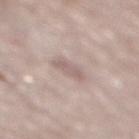patient: male, in their 70s
lesion size: ≈3 mm
imaging modality: ~15 mm tile from a whole-body skin photo
body site: the mid back
image-analysis metrics: a lesion area of about 4 mm², an eccentricity of roughly 0.85, and a shape-asymmetry score of about 0.3 (0 = symmetric); a lesion-detection confidence of about 60/100
illumination: white-light